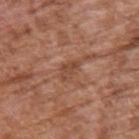{"site": "upper back", "lighting": "white-light", "lesion_size": {"long_diameter_mm_approx": 3.0}, "patient": {"sex": "male", "age_approx": 75}, "image": {"source": "total-body photography crop", "field_of_view_mm": 15}}